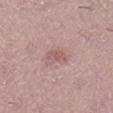notes=imaged on a skin check; not biopsied
lesion size=≈3 mm
imaging modality=~15 mm tile from a whole-body skin photo
lighting=white-light
subject=female, approximately 40 years of age
body site=the right lower leg